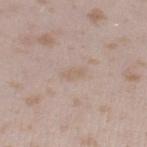Part of a total-body skin-imaging series; this lesion was reviewed on a skin check and was not flagged for biopsy. A roughly 15 mm field-of-view crop from a total-body skin photograph. Captured under white-light illumination. The total-body-photography lesion software estimated a lesion area of about 3 mm². It also reported a mean CIELAB color near L≈62 a*≈14 b*≈26, a lesion–skin lightness drop of about 5, and a lesion-to-skin contrast of about 4.5 (normalized; higher = more distinct). And it measured a border-irregularity index near 2.5/10 and a color-variation rating of about 0.5/10. The software also gave an automated nevus-likeness rating near 0 out of 100. A female subject, roughly 25 years of age. The lesion is on the leg.